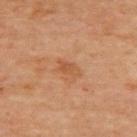notes: total-body-photography surveillance lesion; no biopsy | illumination: cross-polarized | patient: female, aged approximately 70 | diameter: ~2.5 mm (longest diameter) | image: 15 mm crop, total-body photography | body site: the upper back.